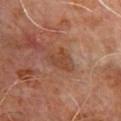Q: Was this lesion biopsied?
A: imaged on a skin check; not biopsied
Q: Where on the body is the lesion?
A: the chest
Q: What did automated image analysis measure?
A: a color-variation rating of about 2/10 and peripheral color asymmetry of about 0.5
Q: What are the patient's age and sex?
A: male, aged around 65
Q: Lesion size?
A: ~3.5 mm (longest diameter)
Q: What is the imaging modality?
A: ~15 mm tile from a whole-body skin photo
Q: Illumination type?
A: cross-polarized illumination